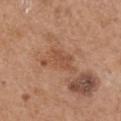Case summary:
– workup · total-body-photography surveillance lesion; no biopsy
– patient · male, in their 70s
– location · the back
– TBP lesion metrics · about 8 CIELAB-L* units darker than the surrounding skin and a normalized border contrast of about 5.5; a classifier nevus-likeness of about 0/100
– acquisition · 15 mm crop, total-body photography